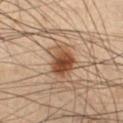The lesion was photographed on a routine skin check and not biopsied; there is no pathology result.
This image is a 15 mm lesion crop taken from a total-body photograph.
A male patient about 55 years old.
Captured under cross-polarized illumination.
Longest diameter approximately 4 mm.
Located on the left lower leg.